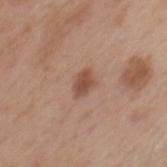notes — no biopsy performed (imaged during a skin exam) | automated metrics — an average lesion color of about L≈50 a*≈21 b*≈29 (CIELAB) and a normalized lesion–skin contrast near 7.5; a border-irregularity rating of about 2.5/10, a within-lesion color-variation index near 2/10, and radial color variation of about 0.5 | location — the chest | image — 15 mm crop, total-body photography | size — about 3 mm | illumination — white-light illumination | subject — female, aged 73–77.